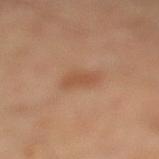Q: Is there a histopathology result?
A: no biopsy performed (imaged during a skin exam)
Q: What did automated image analysis measure?
A: a lesion color around L≈52 a*≈22 b*≈34 in CIELAB, a lesion–skin lightness drop of about 7, and a lesion-to-skin contrast of about 6 (normalized; higher = more distinct); border irregularity of about 2.5 on a 0–10 scale, a within-lesion color-variation index near 1.5/10, and peripheral color asymmetry of about 0.5
Q: What are the patient's age and sex?
A: female, in their 60s
Q: What lighting was used for the tile?
A: cross-polarized illumination
Q: Where on the body is the lesion?
A: the right leg
Q: What is the imaging modality?
A: 15 mm crop, total-body photography
Q: What is the lesion's diameter?
A: about 3 mm